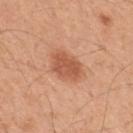Part of a total-body skin-imaging series; this lesion was reviewed on a skin check and was not flagged for biopsy.
A region of skin cropped from a whole-body photographic capture, roughly 15 mm wide.
A male patient about 50 years old.
On the mid back.